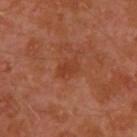Assessment:
Captured during whole-body skin photography for melanoma surveillance; the lesion was not biopsied.
Image and clinical context:
Automated image analysis of the tile measured a lesion area of about 4.5 mm², a shape eccentricity near 0.65, and a symmetry-axis asymmetry near 0.25. And it measured an average lesion color of about L≈39 a*≈27 b*≈33 (CIELAB) and a lesion–skin lightness drop of about 6. It also reported a within-lesion color-variation index near 2.5/10 and peripheral color asymmetry of about 1. The lesion is on the left upper arm. Cropped from a total-body skin-imaging series; the visible field is about 15 mm. Approximately 2.5 mm at its widest. This is a cross-polarized tile. A male subject aged around 30.